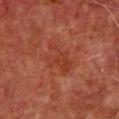Assessment:
Captured during whole-body skin photography for melanoma surveillance; the lesion was not biopsied.
Context:
A roughly 15 mm field-of-view crop from a total-body skin photograph. A male patient roughly 60 years of age. The lesion is located on the chest. Captured under cross-polarized illumination.